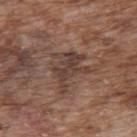Assessment: Recorded during total-body skin imaging; not selected for excision or biopsy. Clinical summary: On the upper back. A male subject aged approximately 75. Cropped from a total-body skin-imaging series; the visible field is about 15 mm.